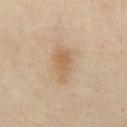Clinical summary:
A close-up tile cropped from a whole-body skin photograph, about 15 mm across. The lesion's longest dimension is about 4.5 mm. The lesion is located on the abdomen. The subject is a male aged 53 to 57. The tile uses cross-polarized illumination.